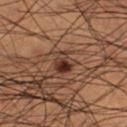This lesion was catalogued during total-body skin photography and was not selected for biopsy. A close-up tile cropped from a whole-body skin photograph, about 15 mm across. Located on the right lower leg. Imaged with cross-polarized lighting. The subject is a male aged 48–52. Automated image analysis of the tile measured a footprint of about 5.5 mm², an outline eccentricity of about 0.5 (0 = round, 1 = elongated), and two-axis asymmetry of about 0.25. And it measured an average lesion color of about L≈26 a*≈16 b*≈21 (CIELAB), roughly 9 lightness units darker than nearby skin, and a lesion-to-skin contrast of about 9.5 (normalized; higher = more distinct). The software also gave a border-irregularity rating of about 2/10. It also reported a detector confidence of about 100 out of 100 that the crop contains a lesion.